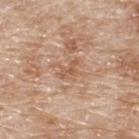Impression:
The lesion was tiled from a total-body skin photograph and was not biopsied.
Background:
A 15 mm crop from a total-body photograph taken for skin-cancer surveillance. The tile uses white-light illumination. From the upper back. A male subject, aged approximately 80. The lesion's longest dimension is about 3 mm.